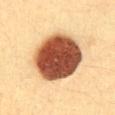{"lesion_size": {"long_diameter_mm_approx": 6.5}, "image": {"source": "total-body photography crop", "field_of_view_mm": 15}, "automated_metrics": {"area_mm2_approx": 33.0, "eccentricity": 0.35, "shape_asymmetry": 0.1, "nevus_likeness_0_100": 100, "lesion_detection_confidence_0_100": 100}, "lighting": "cross-polarized", "site": "abdomen", "patient": {"sex": "female", "age_approx": 30}}